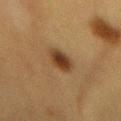Case summary:
* biopsy status: imaged on a skin check; not biopsied
* subject: female, about 55 years old
* location: the mid back
* lighting: cross-polarized
* image source: ~15 mm tile from a whole-body skin photo